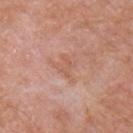biopsy status: catalogued during a skin exam; not biopsied | body site: the arm | patient: male, aged 78–82 | diameter: ≈2.5 mm | automated metrics: a footprint of about 2.5 mm², an outline eccentricity of about 0.9 (0 = round, 1 = elongated), and a symmetry-axis asymmetry near 0.5 | image source: ~15 mm crop, total-body skin-cancer survey | lighting: white-light illumination.